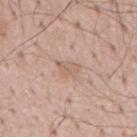biopsy status: catalogued during a skin exam; not biopsied | subject: male, approximately 60 years of age | size: about 2.5 mm | body site: the mid back | imaging modality: total-body-photography crop, ~15 mm field of view.